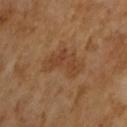{
  "automated_metrics": {
    "area_mm2_approx": 10.0,
    "eccentricity": 0.85,
    "shape_asymmetry": 0.5,
    "border_irregularity_0_10": 6.0,
    "color_variation_0_10": 3.5,
    "peripheral_color_asymmetry": 1.0,
    "nevus_likeness_0_100": 0,
    "lesion_detection_confidence_0_100": 100
  },
  "lesion_size": {
    "long_diameter_mm_approx": 5.0
  },
  "image": {
    "source": "total-body photography crop",
    "field_of_view_mm": 15
  },
  "patient": {
    "sex": "male",
    "age_approx": 65
  },
  "lighting": "cross-polarized"
}